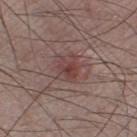Q: Was a biopsy performed?
A: no biopsy performed (imaged during a skin exam)
Q: Who is the patient?
A: male, aged 73 to 77
Q: How was the tile lit?
A: white-light illumination
Q: What kind of image is this?
A: 15 mm crop, total-body photography
Q: Lesion location?
A: the leg
Q: Automated lesion metrics?
A: border irregularity of about 2 on a 0–10 scale, internal color variation of about 5.5 on a 0–10 scale, and peripheral color asymmetry of about 2